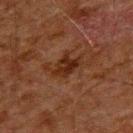Imaged during a routine full-body skin examination; the lesion was not biopsied and no histopathology is available.
On the back.
A male subject, roughly 60 years of age.
This image is a 15 mm lesion crop taken from a total-body photograph.
The recorded lesion diameter is about 3 mm.